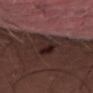Assessment:
Captured during whole-body skin photography for melanoma surveillance; the lesion was not biopsied.